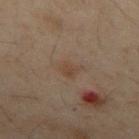<tbp_lesion>
  <biopsy_status>not biopsied; imaged during a skin examination</biopsy_status>
  <site>mid back</site>
  <automated_metrics>
    <area_mm2_approx>4.5</area_mm2_approx>
    <eccentricity>0.55</eccentricity>
    <shape_asymmetry>0.3</shape_asymmetry>
    <cielab_L>32</cielab_L>
    <cielab_a>11</cielab_a>
    <cielab_b>21</cielab_b>
    <vs_skin_darker_L>4.0</vs_skin_darker_L>
    <vs_skin_contrast_norm>5.0</vs_skin_contrast_norm>
    <border_irregularity_0_10>3.0</border_irregularity_0_10>
    <peripheral_color_asymmetry>0.5</peripheral_color_asymmetry>
  </automated_metrics>
  <image>
    <source>total-body photography crop</source>
    <field_of_view_mm>15</field_of_view_mm>
  </image>
  <patient>
    <sex>male</sex>
    <age_approx>50</age_approx>
  </patient>
  <lesion_size>
    <long_diameter_mm_approx>2.5</long_diameter_mm_approx>
  </lesion_size>
</tbp_lesion>